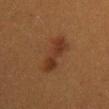Notes:
• workup: total-body-photography surveillance lesion; no biopsy
• location: the mid back
• acquisition: ~15 mm crop, total-body skin-cancer survey
• patient: female, roughly 40 years of age